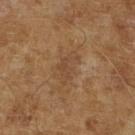Case summary:
* image source: ~15 mm tile from a whole-body skin photo
* size: ≈4 mm
* lighting: cross-polarized illumination
* patient: male, aged approximately 65